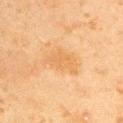  biopsy_status: not biopsied; imaged during a skin examination
  image:
    source: total-body photography crop
    field_of_view_mm: 15
  automated_metrics:
    area_mm2_approx: 8.0
    eccentricity: 0.85
    shape_asymmetry: 0.4
    color_variation_0_10: 2.0
    peripheral_color_asymmetry: 0.5
  patient:
    sex: male
    age_approx: 45
  site: right upper arm
  lesion_size:
    long_diameter_mm_approx: 4.5
  lighting: cross-polarized